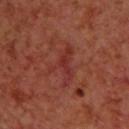Clinical impression: The lesion was photographed on a routine skin check and not biopsied; there is no pathology result. Image and clinical context: The lesion is on the upper back. A male patient aged 68–72. Cropped from a whole-body photographic skin survey; the tile spans about 15 mm.